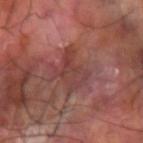{
  "biopsy_status": "not biopsied; imaged during a skin examination",
  "lesion_size": {
    "long_diameter_mm_approx": 4.5
  },
  "automated_metrics": {
    "area_mm2_approx": 11.0,
    "eccentricity": 0.65,
    "shape_asymmetry": 0.35,
    "cielab_L": 39,
    "cielab_a": 24,
    "cielab_b": 22,
    "vs_skin_darker_L": 7.0,
    "vs_skin_contrast_norm": 5.5
  },
  "image": {
    "source": "total-body photography crop",
    "field_of_view_mm": 15
  },
  "patient": {
    "sex": "male",
    "age_approx": 60
  },
  "lighting": "cross-polarized",
  "site": "left forearm"
}